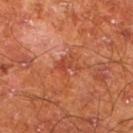biopsy status — total-body-photography surveillance lesion; no biopsy | acquisition — ~15 mm crop, total-body skin-cancer survey | subject — male, aged around 65 | anatomic site — the left lower leg.